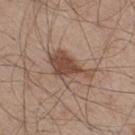| key | value |
|---|---|
| workup | catalogued during a skin exam; not biopsied |
| body site | the right thigh |
| acquisition | 15 mm crop, total-body photography |
| lesion diameter | about 5.5 mm |
| patient | male, aged around 45 |
| lighting | white-light illumination |
| TBP lesion metrics | a footprint of about 12 mm², a shape eccentricity near 0.8, and a shape-asymmetry score of about 0.45 (0 = symmetric); about 11 CIELAB-L* units darker than the surrounding skin; a border-irregularity index near 5.5/10 and radial color variation of about 1.5; a lesion-detection confidence of about 100/100 |